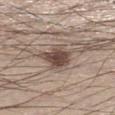Clinical impression: Recorded during total-body skin imaging; not selected for excision or biopsy. Image and clinical context: A 15 mm close-up extracted from a 3D total-body photography capture. Automated image analysis of the tile measured a lesion-to-skin contrast of about 9.5 (normalized; higher = more distinct). And it measured a border-irregularity rating of about 3.5/10, a within-lesion color-variation index near 4/10, and a peripheral color-asymmetry measure near 1. A male subject, roughly 55 years of age. On the left lower leg. The tile uses white-light illumination. About 4.5 mm across.